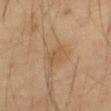Findings:
– follow-up: catalogued during a skin exam; not biopsied
– imaging modality: ~15 mm crop, total-body skin-cancer survey
– body site: the right thigh
– subject: male, aged 68 to 72